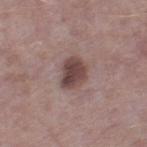No biopsy was performed on this lesion — it was imaged during a full skin examination and was not determined to be concerning. A male subject in their mid- to late 60s. This is a white-light tile. On the leg. Approximately 3.5 mm at its widest. Cropped from a whole-body photographic skin survey; the tile spans about 15 mm.